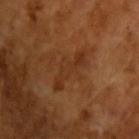| field | value |
|---|---|
| biopsy status | no biopsy performed (imaged during a skin exam) |
| illumination | cross-polarized illumination |
| acquisition | 15 mm crop, total-body photography |
| automated metrics | an area of roughly 8 mm², an outline eccentricity of about 0.95 (0 = round, 1 = elongated), and a symmetry-axis asymmetry near 0.45; a lesion color around L≈32 a*≈22 b*≈32 in CIELAB; a classifier nevus-likeness of about 0/100 |
| patient | male, in their mid- to late 60s |